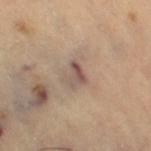Clinical impression: No biopsy was performed on this lesion — it was imaged during a full skin examination and was not determined to be concerning. Context: Imaged with cross-polarized lighting. The lesion's longest dimension is about 3 mm. The lesion is located on the leg. Cropped from a whole-body photographic skin survey; the tile spans about 15 mm. The patient is a female approximately 70 years of age.